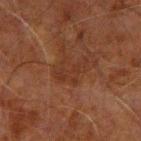| key | value |
|---|---|
| biopsy status | catalogued during a skin exam; not biopsied |
| image source | total-body-photography crop, ~15 mm field of view |
| location | the left arm |
| automated metrics | an average lesion color of about L≈25 a*≈18 b*≈24 (CIELAB), about 4 CIELAB-L* units darker than the surrounding skin, and a normalized border contrast of about 5; a border-irregularity index near 5/10; a classifier nevus-likeness of about 0/100 and a lesion-detection confidence of about 100/100 |
| patient | male, aged 58–62 |
| lighting | cross-polarized |